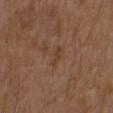Clinical impression: This lesion was catalogued during total-body skin photography and was not selected for biopsy. Acquisition and patient details: The lesion is located on the upper back. A region of skin cropped from a whole-body photographic capture, roughly 15 mm wide. About 2.5 mm across. A male subject, about 75 years old.